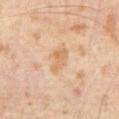The lesion was photographed on a routine skin check and not biopsied; there is no pathology result.
A region of skin cropped from a whole-body photographic capture, roughly 15 mm wide.
Measured at roughly 3 mm in maximum diameter.
A male patient in their mid-60s.